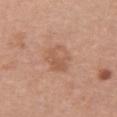This lesion was catalogued during total-body skin photography and was not selected for biopsy.
The recorded lesion diameter is about 3.5 mm.
The tile uses white-light illumination.
Cropped from a total-body skin-imaging series; the visible field is about 15 mm.
A female subject, roughly 60 years of age.
On the chest.